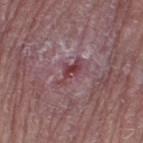workup — imaged on a skin check; not biopsied
lighting — white-light illumination
acquisition — ~15 mm crop, total-body skin-cancer survey
lesion size — about 3.5 mm
anatomic site — the right thigh
subject — male, approximately 65 years of age